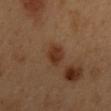Findings:
* notes · catalogued during a skin exam; not biopsied
* subject · male, roughly 50 years of age
* lesion size · about 3 mm
* imaging modality · 15 mm crop, total-body photography
* anatomic site · the mid back